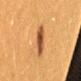Background:
Approximately 3.5 mm at its widest. From the back. Cropped from a total-body skin-imaging series; the visible field is about 15 mm. A female subject about 40 years old.